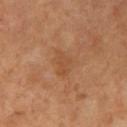The lesion was photographed on a routine skin check and not biopsied; there is no pathology result. Located on the right thigh. Measured at roughly 4 mm in maximum diameter. The total-body-photography lesion software estimated an area of roughly 7 mm², an eccentricity of roughly 0.65, and two-axis asymmetry of about 0.35. It also reported a lesion color around L≈51 a*≈23 b*≈36 in CIELAB and a lesion-to-skin contrast of about 4.5 (normalized; higher = more distinct). The analysis additionally found border irregularity of about 4 on a 0–10 scale and peripheral color asymmetry of about 1. The tile uses cross-polarized illumination. A female patient, about 55 years old. A close-up tile cropped from a whole-body skin photograph, about 15 mm across.